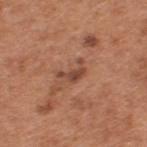| key | value |
|---|---|
| workup | no biopsy performed (imaged during a skin exam) |
| automated lesion analysis | a lesion color around L≈46 a*≈23 b*≈29 in CIELAB, roughly 10 lightness units darker than nearby skin, and a normalized lesion–skin contrast near 7.5; internal color variation of about 1 on a 0–10 scale and a peripheral color-asymmetry measure near 0.5; a classifier nevus-likeness of about 0/100 and a detector confidence of about 100 out of 100 that the crop contains a lesion |
| patient | male, aged 63–67 |
| size | about 3 mm |
| illumination | white-light |
| acquisition | ~15 mm tile from a whole-body skin photo |
| anatomic site | the back |